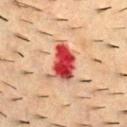Q: Was a biopsy performed?
A: no biopsy performed (imaged during a skin exam)
Q: What is the lesion's diameter?
A: ≈5 mm
Q: Who is the patient?
A: male, aged approximately 55
Q: Lesion location?
A: the upper back
Q: What lighting was used for the tile?
A: cross-polarized
Q: What kind of image is this?
A: total-body-photography crop, ~15 mm field of view